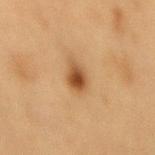No biopsy was performed on this lesion — it was imaged during a full skin examination and was not determined to be concerning. An algorithmic analysis of the crop reported an area of roughly 5.5 mm², a shape eccentricity near 0.75, and a shape-asymmetry score of about 0.2 (0 = symmetric). And it measured a mean CIELAB color near L≈44 a*≈19 b*≈34, about 12 CIELAB-L* units darker than the surrounding skin, and a lesion-to-skin contrast of about 9.5 (normalized; higher = more distinct). The software also gave a classifier nevus-likeness of about 100/100 and lesion-presence confidence of about 100/100. A female patient aged 38–42. The lesion is on the lower back. Measured at roughly 3.5 mm in maximum diameter. Cropped from a total-body skin-imaging series; the visible field is about 15 mm. The tile uses cross-polarized illumination.